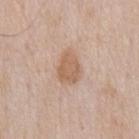The subject is a male about 60 years old. From the front of the torso. A lesion tile, about 15 mm wide, cut from a 3D total-body photograph.